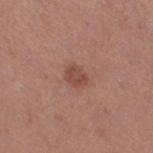Q: Is there a histopathology result?
A: catalogued during a skin exam; not biopsied
Q: Automated lesion metrics?
A: an outline eccentricity of about 0.65 (0 = round, 1 = elongated); a normalized lesion–skin contrast near 6.5; a border-irregularity index near 2/10 and internal color variation of about 1.5 on a 0–10 scale
Q: What is the imaging modality?
A: total-body-photography crop, ~15 mm field of view
Q: What is the anatomic site?
A: the right thigh
Q: How large is the lesion?
A: ≈2.5 mm
Q: Illumination type?
A: white-light
Q: What are the patient's age and sex?
A: female, aged 38 to 42